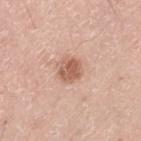| feature | finding |
|---|---|
| biopsy status | total-body-photography surveillance lesion; no biopsy |
| patient | male, aged approximately 50 |
| site | the left thigh |
| acquisition | ~15 mm crop, total-body skin-cancer survey |
| lesion diameter | ≈3 mm |
| tile lighting | white-light |